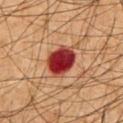Part of a total-body skin-imaging series; this lesion was reviewed on a skin check and was not flagged for biopsy. Automated image analysis of the tile measured a footprint of about 11 mm², a shape eccentricity near 0.7, and a shape-asymmetry score of about 0.2 (0 = symmetric). The recorded lesion diameter is about 4.5 mm. On the chest. This is a cross-polarized tile. A male subject about 65 years old. A 15 mm close-up extracted from a 3D total-body photography capture.